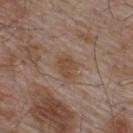notes: no biopsy performed (imaged during a skin exam).